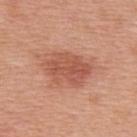follow-up = no biopsy performed (imaged during a skin exam)
subject = female, aged 38–42
body site = the upper back
automated lesion analysis = an area of roughly 14 mm², a shape eccentricity near 0.75, and two-axis asymmetry of about 0.25; a classifier nevus-likeness of about 80/100 and a detector confidence of about 100 out of 100 that the crop contains a lesion
acquisition = 15 mm crop, total-body photography
size = about 5 mm